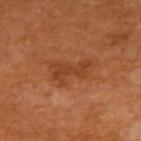follow-up: imaged on a skin check; not biopsied
anatomic site: the upper back
subject: female, aged around 55
image: ~15 mm crop, total-body skin-cancer survey
image-analysis metrics: a footprint of about 8 mm², a shape eccentricity near 0.9, and two-axis asymmetry of about 0.35; a lesion color around L≈43 a*≈27 b*≈39 in CIELAB and roughly 8 lightness units darker than nearby skin
illumination: cross-polarized illumination
lesion diameter: ~4.5 mm (longest diameter)